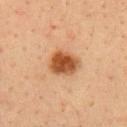Impression:
The lesion was tiled from a total-body skin photograph and was not biopsied.
Acquisition and patient details:
This is a cross-polarized tile. The lesion is on the upper back. A 15 mm crop from a total-body photograph taken for skin-cancer surveillance. A male subject, approximately 35 years of age. The total-body-photography lesion software estimated a lesion color around L≈49 a*≈24 b*≈37 in CIELAB, about 15 CIELAB-L* units darker than the surrounding skin, and a normalized border contrast of about 11. It also reported a border-irregularity index near 1/10, a color-variation rating of about 4.5/10, and peripheral color asymmetry of about 1.5. It also reported a nevus-likeness score of about 100/100 and a lesion-detection confidence of about 100/100.